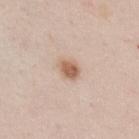Q: Was a biopsy performed?
A: catalogued during a skin exam; not biopsied
Q: Who is the patient?
A: male, in their 50s
Q: Lesion size?
A: about 2.5 mm
Q: Illumination type?
A: white-light
Q: What is the imaging modality?
A: 15 mm crop, total-body photography
Q: What is the anatomic site?
A: the chest